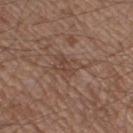Case summary:
- notes — imaged on a skin check; not biopsied
- image — ~15 mm tile from a whole-body skin photo
- location — the leg
- lesion size — about 3 mm
- image-analysis metrics — a lesion area of about 6 mm² and a shape-asymmetry score of about 0.4 (0 = symmetric); a lesion color around L≈44 a*≈18 b*≈25 in CIELAB, about 6 CIELAB-L* units darker than the surrounding skin, and a normalized border contrast of about 5; a within-lesion color-variation index near 3/10 and peripheral color asymmetry of about 1
- subject — male, aged 53–57
- tile lighting — white-light